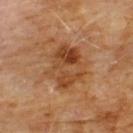Assessment:
Part of a total-body skin-imaging series; this lesion was reviewed on a skin check and was not flagged for biopsy.
Image and clinical context:
Approximately 5.5 mm at its widest. A 15 mm close-up extracted from a 3D total-body photography capture. The subject is a male about 60 years old. This is a cross-polarized tile. The lesion is on the chest.